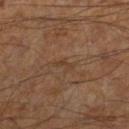No biopsy was performed on this lesion — it was imaged during a full skin examination and was not determined to be concerning.